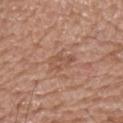Impression: Imaged during a routine full-body skin examination; the lesion was not biopsied and no histopathology is available. Background: A male patient in their mid- to late 70s. This image is a 15 mm lesion crop taken from a total-body photograph. The recorded lesion diameter is about 3 mm. The tile uses white-light illumination. On the upper back. The total-body-photography lesion software estimated a border-irregularity rating of about 4/10, a color-variation rating of about 3.5/10, and a peripheral color-asymmetry measure near 1.5.